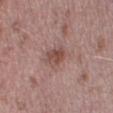biopsy_status: not biopsied; imaged during a skin examination
automated_metrics:
  area_mm2_approx: 5.5
  eccentricity: 0.65
  lesion_detection_confidence_0_100: 100
image:
  source: total-body photography crop
  field_of_view_mm: 15
patient:
  sex: male
  age_approx: 40
site: left lower leg